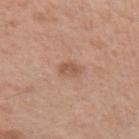Q: Was a biopsy performed?
A: total-body-photography surveillance lesion; no biopsy
Q: Who is the patient?
A: male, in their mid- to late 40s
Q: Lesion location?
A: the left upper arm
Q: What is the imaging modality?
A: total-body-photography crop, ~15 mm field of view
Q: What lighting was used for the tile?
A: white-light illumination
Q: What did automated image analysis measure?
A: a footprint of about 3.5 mm², an eccentricity of roughly 0.7, and two-axis asymmetry of about 0.3; about 8 CIELAB-L* units darker than the surrounding skin and a lesion-to-skin contrast of about 6 (normalized; higher = more distinct); a nevus-likeness score of about 5/100 and a detector confidence of about 100 out of 100 that the crop contains a lesion
Q: How large is the lesion?
A: about 2.5 mm